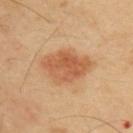Clinical impression:
Part of a total-body skin-imaging series; this lesion was reviewed on a skin check and was not flagged for biopsy.
Background:
The lesion is located on the back. A 15 mm close-up tile from a total-body photography series done for melanoma screening. The lesion-visualizer software estimated a lesion color around L≈56 a*≈23 b*≈37 in CIELAB, about 11 CIELAB-L* units darker than the surrounding skin, and a lesion-to-skin contrast of about 7.5 (normalized; higher = more distinct). And it measured a within-lesion color-variation index near 4/10 and radial color variation of about 1.5. A male subject about 50 years old.